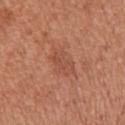No biopsy was performed on this lesion — it was imaged during a full skin examination and was not determined to be concerning.
A female patient, about 65 years old.
The lesion-visualizer software estimated a lesion area of about 8 mm², an eccentricity of roughly 0.8, and a shape-asymmetry score of about 0.2 (0 = symmetric). The software also gave an average lesion color of about L≈51 a*≈25 b*≈32 (CIELAB) and about 6 CIELAB-L* units darker than the surrounding skin.
Located on the arm.
A 15 mm close-up tile from a total-body photography series done for melanoma screening.
Approximately 4.5 mm at its widest.